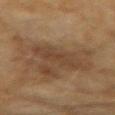{"biopsy_status": "not biopsied; imaged during a skin examination", "image": {"source": "total-body photography crop", "field_of_view_mm": 15}, "lighting": "cross-polarized", "site": "arm", "patient": {"sex": "female", "age_approx": 60}}